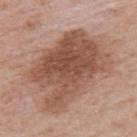Part of a total-body skin-imaging series; this lesion was reviewed on a skin check and was not flagged for biopsy.
A female subject, roughly 60 years of age.
A roughly 15 mm field-of-view crop from a total-body skin photograph.
The tile uses white-light illumination.
The lesion is located on the upper back.